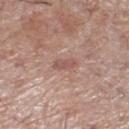No biopsy was performed on this lesion — it was imaged during a full skin examination and was not determined to be concerning.
A male patient, aged around 70.
The lesion is located on the right lower leg.
A 15 mm close-up extracted from a 3D total-body photography capture.